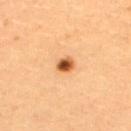biopsy status — imaged on a skin check; not biopsied
patient — female, aged 28 to 32
tile lighting — cross-polarized
anatomic site — the upper back
automated metrics — a color-variation rating of about 5.5/10 and a peripheral color-asymmetry measure near 1.5
diameter — ≈2 mm
acquisition — total-body-photography crop, ~15 mm field of view